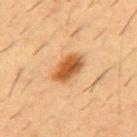Captured during whole-body skin photography for melanoma surveillance; the lesion was not biopsied.
A 15 mm crop from a total-body photograph taken for skin-cancer surveillance.
Automated image analysis of the tile measured a mean CIELAB color near L≈50 a*≈22 b*≈38 and a lesion–skin lightness drop of about 13. And it measured internal color variation of about 5 on a 0–10 scale and a peripheral color-asymmetry measure near 1.5.
On the chest.
Approximately 4.5 mm at its widest.
Imaged with cross-polarized lighting.
A male subject aged 53 to 57.